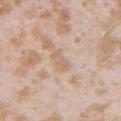Acquisition and patient details: The lesion is on the left upper arm. A close-up tile cropped from a whole-body skin photograph, about 15 mm across. A female patient, aged 23–27. Approximately 2.5 mm at its widest. This is a white-light tile. The lesion-visualizer software estimated a border-irregularity rating of about 2.5/10 and a peripheral color-asymmetry measure near 0.5. And it measured a classifier nevus-likeness of about 0/100 and a lesion-detection confidence of about 90/100.